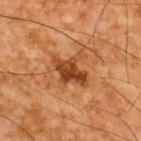Notes:
• notes · catalogued during a skin exam; not biopsied
• acquisition · ~15 mm crop, total-body skin-cancer survey
• automated metrics · an area of roughly 10 mm², an outline eccentricity of about 0.7 (0 = round, 1 = elongated), and two-axis asymmetry of about 0.3; an average lesion color of about L≈35 a*≈23 b*≈33 (CIELAB) and a lesion–skin lightness drop of about 10; border irregularity of about 5 on a 0–10 scale and a within-lesion color-variation index near 4/10
• illumination · cross-polarized illumination
• location · the upper back
• patient · male, in their mid-60s